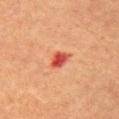From the chest.
The tile uses cross-polarized illumination.
The recorded lesion diameter is about 2.5 mm.
A lesion tile, about 15 mm wide, cut from a 3D total-body photograph.
A female subject, in their 70s.